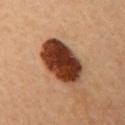Impression:
No biopsy was performed on this lesion — it was imaged during a full skin examination and was not determined to be concerning.